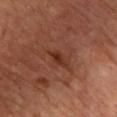follow-up: total-body-photography surveillance lesion; no biopsy | imaging modality: ~15 mm tile from a whole-body skin photo | automated lesion analysis: an area of roughly 3.5 mm² and an eccentricity of roughly 0.9; an average lesion color of about L≈29 a*≈23 b*≈27 (CIELAB), about 8 CIELAB-L* units darker than the surrounding skin, and a lesion-to-skin contrast of about 8 (normalized; higher = more distinct); a nevus-likeness score of about 25/100 and lesion-presence confidence of about 100/100 | lesion diameter: about 3.5 mm | patient: male, in their mid-60s | location: the upper back | lighting: cross-polarized.